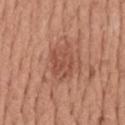{"biopsy_status": "not biopsied; imaged during a skin examination", "automated_metrics": {"cielab_L": 51, "cielab_a": 25, "cielab_b": 30, "vs_skin_darker_L": 8.0, "border_irregularity_0_10": 3.0, "color_variation_0_10": 3.0, "peripheral_color_asymmetry": 1.0}, "image": {"source": "total-body photography crop", "field_of_view_mm": 15}, "lesion_size": {"long_diameter_mm_approx": 4.0}, "patient": {"sex": "male", "age_approx": 55}, "site": "mid back"}